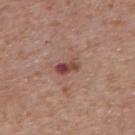Clinical impression:
Recorded during total-body skin imaging; not selected for excision or biopsy.
Image and clinical context:
A region of skin cropped from a whole-body photographic capture, roughly 15 mm wide. From the mid back. The subject is a male aged approximately 70.